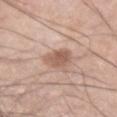{"biopsy_status": "not biopsied; imaged during a skin examination", "patient": {"sex": "male", "age_approx": 65}, "lesion_size": {"long_diameter_mm_approx": 3.5}, "automated_metrics": {"border_irregularity_0_10": 2.5, "color_variation_0_10": 3.0, "peripheral_color_asymmetry": 1.0, "nevus_likeness_0_100": 40, "lesion_detection_confidence_0_100": 100}, "site": "left upper arm", "image": {"source": "total-body photography crop", "field_of_view_mm": 15}}